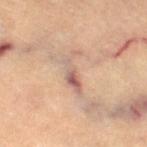* notes: total-body-photography surveillance lesion; no biopsy
* lighting: cross-polarized illumination
* automated lesion analysis: a footprint of about 6 mm², an eccentricity of roughly 0.9, and a shape-asymmetry score of about 0.65 (0 = symmetric)
* imaging modality: 15 mm crop, total-body photography
* subject: female, in their mid-60s
* body site: the left leg